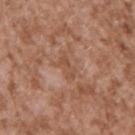{
  "biopsy_status": "not biopsied; imaged during a skin examination",
  "patient": {
    "sex": "male",
    "age_approx": 45
  },
  "lighting": "white-light",
  "image": {
    "source": "total-body photography crop",
    "field_of_view_mm": 15
  },
  "site": "right upper arm"
}